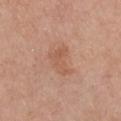Q: Is there a histopathology result?
A: catalogued during a skin exam; not biopsied
Q: What is the imaging modality?
A: total-body-photography crop, ~15 mm field of view
Q: What are the patient's age and sex?
A: female, aged around 55
Q: What is the anatomic site?
A: the chest
Q: What lighting was used for the tile?
A: white-light